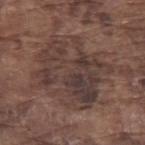The recorded lesion diameter is about 8 mm. The tile uses white-light illumination. This image is a 15 mm lesion crop taken from a total-body photograph. A male subject, aged 73–77. The total-body-photography lesion software estimated border irregularity of about 7.5 on a 0–10 scale, internal color variation of about 6.5 on a 0–10 scale, and peripheral color asymmetry of about 2. Located on the arm.